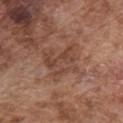A male subject aged approximately 75. On the chest. Imaged with white-light lighting. The recorded lesion diameter is about 4.5 mm. This image is a 15 mm lesion crop taken from a total-body photograph.